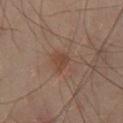  biopsy_status: not biopsied; imaged during a skin examination
  image:
    source: total-body photography crop
    field_of_view_mm: 15
  lighting: cross-polarized
  patient:
    sex: male
    age_approx: 50
  site: leg
  lesion_size:
    long_diameter_mm_approx: 2.5